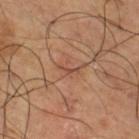Impression:
The lesion was tiled from a total-body skin photograph and was not biopsied.
Context:
A region of skin cropped from a whole-body photographic capture, roughly 15 mm wide. A male patient, aged around 65. Measured at roughly 2.5 mm in maximum diameter. Imaged with cross-polarized lighting. The lesion is located on the leg.